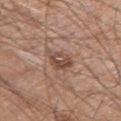This lesion was catalogued during total-body skin photography and was not selected for biopsy.
A male subject in their mid- to late 60s.
An algorithmic analysis of the crop reported a nevus-likeness score of about 35/100 and a detector confidence of about 100 out of 100 that the crop contains a lesion.
This is a white-light tile.
Longest diameter approximately 3.5 mm.
The lesion is on the arm.
A region of skin cropped from a whole-body photographic capture, roughly 15 mm wide.